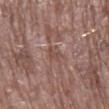<record>
  <biopsy_status>not biopsied; imaged during a skin examination</biopsy_status>
  <lighting>white-light</lighting>
  <site>right lower leg</site>
  <patient>
    <sex>male</sex>
    <age_approx>80</age_approx>
  </patient>
  <image>
    <source>total-body photography crop</source>
    <field_of_view_mm>15</field_of_view_mm>
  </image>
  <lesion_size>
    <long_diameter_mm_approx>2.5</long_diameter_mm_approx>
  </lesion_size>
</record>